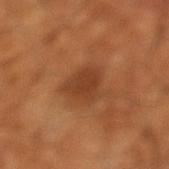Clinical impression:
Part of a total-body skin-imaging series; this lesion was reviewed on a skin check and was not flagged for biopsy.
Clinical summary:
Imaged with cross-polarized lighting. A close-up tile cropped from a whole-body skin photograph, about 15 mm across. The lesion's longest dimension is about 4.5 mm. The lesion is on the left lower leg. A male subject, aged 63 to 67.